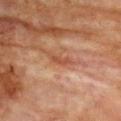This lesion was catalogued during total-body skin photography and was not selected for biopsy.
The lesion-visualizer software estimated a lesion color around L≈43 a*≈23 b*≈30 in CIELAB and about 8 CIELAB-L* units darker than the surrounding skin. The analysis additionally found a border-irregularity rating of about 4.5/10, internal color variation of about 0 on a 0–10 scale, and a peripheral color-asymmetry measure near 0. And it measured a lesion-detection confidence of about 55/100.
A roughly 15 mm field-of-view crop from a total-body skin photograph.
Located on the upper back.
A female patient, in their 80s.